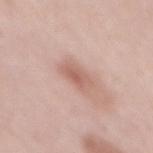Q: Is there a histopathology result?
A: total-body-photography surveillance lesion; no biopsy
Q: Illumination type?
A: white-light illumination
Q: Automated lesion metrics?
A: an eccentricity of roughly 0.8 and two-axis asymmetry of about 0.25; a classifier nevus-likeness of about 50/100 and a lesion-detection confidence of about 100/100
Q: What is the anatomic site?
A: the mid back
Q: Patient demographics?
A: female, approximately 60 years of age
Q: Lesion size?
A: about 3 mm
Q: What is the imaging modality?
A: 15 mm crop, total-body photography